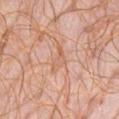<record>
<biopsy_status>not biopsied; imaged during a skin examination</biopsy_status>
<lesion_size>
  <long_diameter_mm_approx>3.5</long_diameter_mm_approx>
</lesion_size>
<image>
  <source>total-body photography crop</source>
  <field_of_view_mm>15</field_of_view_mm>
</image>
<patient>
  <sex>female</sex>
  <age_approx>45</age_approx>
</patient>
<site>front of the torso</site>
<lighting>white-light</lighting>
<automated_metrics>
  <border_irregularity_0_10>6.5</border_irregularity_0_10>
  <color_variation_0_10>1.5</color_variation_0_10>
  <peripheral_color_asymmetry>0.5</peripheral_color_asymmetry>
  <lesion_detection_confidence_0_100>95</lesion_detection_confidence_0_100>
</automated_metrics>
</record>